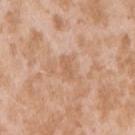Impression:
The lesion was photographed on a routine skin check and not biopsied; there is no pathology result.
Context:
About 2.5 mm across. Located on the right upper arm. A female patient, aged approximately 25. A region of skin cropped from a whole-body photographic capture, roughly 15 mm wide. The lesion-visualizer software estimated a footprint of about 3.5 mm², an eccentricity of roughly 0.85, and a symmetry-axis asymmetry near 0.25.